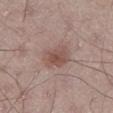Assessment:
Captured during whole-body skin photography for melanoma surveillance; the lesion was not biopsied.
Acquisition and patient details:
About 3.5 mm across. The lesion-visualizer software estimated an area of roughly 8.5 mm² and two-axis asymmetry of about 0.25. And it measured border irregularity of about 2.5 on a 0–10 scale, internal color variation of about 3.5 on a 0–10 scale, and a peripheral color-asymmetry measure near 1. Captured under white-light illumination. The patient is a male aged approximately 65. A 15 mm crop from a total-body photograph taken for skin-cancer surveillance. On the right thigh.